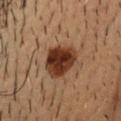Background: Approximately 5 mm at its widest. A roughly 15 mm field-of-view crop from a total-body skin photograph. A male subject roughly 50 years of age. Located on the head or neck. This is a cross-polarized tile.